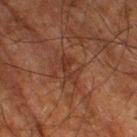  biopsy_status: not biopsied; imaged during a skin examination
  site: right thigh
  lesion_size:
    long_diameter_mm_approx: 3.5
  lighting: cross-polarized
  image:
    source: total-body photography crop
    field_of_view_mm: 15
  automated_metrics:
    area_mm2_approx: 4.0
    eccentricity: 0.9
    shape_asymmetry: 0.4
    cielab_L: 26
    cielab_a: 19
    cielab_b: 23
    vs_skin_contrast_norm: 6.0
  patient:
    sex: male
    age_approx: 80